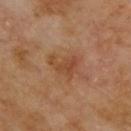Q: Was a biopsy performed?
A: imaged on a skin check; not biopsied
Q: Who is the patient?
A: male, aged approximately 70
Q: What is the imaging modality?
A: 15 mm crop, total-body photography
Q: Lesion size?
A: ~3.5 mm (longest diameter)
Q: What is the anatomic site?
A: the upper back
Q: What lighting was used for the tile?
A: cross-polarized illumination